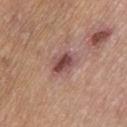Impression:
This lesion was catalogued during total-body skin photography and was not selected for biopsy.
Image and clinical context:
A roughly 15 mm field-of-view crop from a total-body skin photograph. The lesion-visualizer software estimated a lesion area of about 8.5 mm² and two-axis asymmetry of about 0.25. The analysis additionally found an automated nevus-likeness rating near 10 out of 100. Located on the left thigh. A female subject, aged 58 to 62. This is a white-light tile.